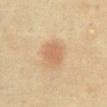anatomic site: the chest | image: total-body-photography crop, ~15 mm field of view | subject: female, aged 58 to 62 | lighting: cross-polarized illumination | image-analysis metrics: a shape eccentricity near 0.35 and a symmetry-axis asymmetry near 0.15; about 8 CIELAB-L* units darker than the surrounding skin; border irregularity of about 1.5 on a 0–10 scale, internal color variation of about 1.5 on a 0–10 scale, and radial color variation of about 0.5; an automated nevus-likeness rating near 65 out of 100 | lesion diameter: about 3 mm.